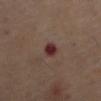Impression: Captured during whole-body skin photography for melanoma surveillance; the lesion was not biopsied. Background: Captured under cross-polarized illumination. A female subject, in their 40s. The recorded lesion diameter is about 2.5 mm. A 15 mm close-up extracted from a 3D total-body photography capture. The lesion is on the right thigh.